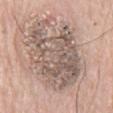This lesion was catalogued during total-body skin photography and was not selected for biopsy. Cropped from a whole-body photographic skin survey; the tile spans about 15 mm. A male subject, about 80 years old. The lesion is on the back.